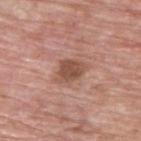  biopsy_status: not biopsied; imaged during a skin examination
  patient:
    sex: male
    age_approx: 65
  image:
    source: total-body photography crop
    field_of_view_mm: 15
  lesion_size:
    long_diameter_mm_approx: 3.5
  lighting: white-light
  site: upper back